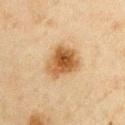workup: no biopsy performed (imaged during a skin exam)
imaging modality: ~15 mm crop, total-body skin-cancer survey
location: the arm
subject: male, in their mid- to late 40s
size: ≈4.5 mm